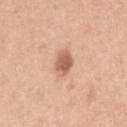follow-up = imaged on a skin check; not biopsied | body site = the right upper arm | acquisition = ~15 mm crop, total-body skin-cancer survey | lighting = white-light | patient = female, in their 40s.